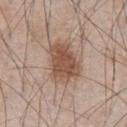From the chest.
A male subject, aged 63 to 67.
A 15 mm close-up extracted from a 3D total-body photography capture.